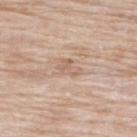Assessment: Imaged during a routine full-body skin examination; the lesion was not biopsied and no histopathology is available. Clinical summary: The subject is a male in their mid- to late 70s. The lesion is on the back. An algorithmic analysis of the crop reported a border-irregularity rating of about 4.5/10, internal color variation of about 1.5 on a 0–10 scale, and peripheral color asymmetry of about 0.5. The software also gave a classifier nevus-likeness of about 0/100 and a detector confidence of about 90 out of 100 that the crop contains a lesion. A 15 mm close-up tile from a total-body photography series done for melanoma screening.